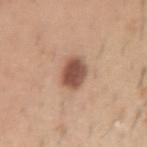biopsy status: imaged on a skin check; not biopsied | image source: 15 mm crop, total-body photography | patient: male, aged 58–62 | lesion size: ~3.5 mm (longest diameter) | automated metrics: an average lesion color of about L≈51 a*≈21 b*≈28 (CIELAB) and a lesion–skin lightness drop of about 16; a border-irregularity index near 1.5/10, a within-lesion color-variation index near 4/10, and a peripheral color-asymmetry measure near 1; a classifier nevus-likeness of about 95/100 and a lesion-detection confidence of about 100/100 | anatomic site: the right upper arm | illumination: white-light.